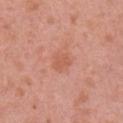Q: Was this lesion biopsied?
A: total-body-photography surveillance lesion; no biopsy
Q: What lighting was used for the tile?
A: white-light illumination
Q: What is the anatomic site?
A: the right upper arm
Q: What kind of image is this?
A: total-body-photography crop, ~15 mm field of view
Q: Who is the patient?
A: female, aged around 40